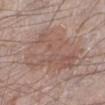<lesion>
<biopsy_status>not biopsied; imaged during a skin examination</biopsy_status>
<lesion_size>
  <long_diameter_mm_approx>9.0</long_diameter_mm_approx>
</lesion_size>
<site>left lower leg</site>
<patient>
  <sex>male</sex>
  <age_approx>60</age_approx>
</patient>
<image>
  <source>total-body photography crop</source>
  <field_of_view_mm>15</field_of_view_mm>
</image>
<automated_metrics>
  <cielab_L>55</cielab_L>
  <cielab_a>18</cielab_a>
  <cielab_b>24</cielab_b>
  <vs_skin_darker_L>7.0</vs_skin_darker_L>
  <vs_skin_contrast_norm>5.0</vs_skin_contrast_norm>
</automated_metrics>
</lesion>